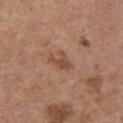Impression:
The lesion was tiled from a total-body skin photograph and was not biopsied.
Acquisition and patient details:
The lesion is located on the front of the torso. A female patient, in their mid- to late 60s. A region of skin cropped from a whole-body photographic capture, roughly 15 mm wide. Imaged with white-light lighting.